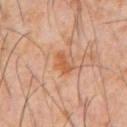{
  "biopsy_status": "not biopsied; imaged during a skin examination",
  "lesion_size": {
    "long_diameter_mm_approx": 3.0
  },
  "image": {
    "source": "total-body photography crop",
    "field_of_view_mm": 15
  },
  "automated_metrics": {
    "vs_skin_contrast_norm": 7.0,
    "nevus_likeness_0_100": 0
  },
  "patient": {
    "sex": "male",
    "age_approx": 60
  },
  "lighting": "cross-polarized",
  "site": "abdomen"
}